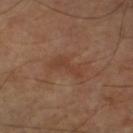Assessment: Captured during whole-body skin photography for melanoma surveillance; the lesion was not biopsied. Image and clinical context: About 4 mm across. The lesion is on the arm. A male patient, in their 60s. Imaged with cross-polarized lighting. A lesion tile, about 15 mm wide, cut from a 3D total-body photograph.